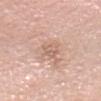Findings:
- tile lighting: white-light
- image source: ~15 mm crop, total-body skin-cancer survey
- subject: female, aged approximately 60
- body site: the head or neck